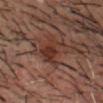This lesion was catalogued during total-body skin photography and was not selected for biopsy.
Automated tile analysis of the lesion measured a detector confidence of about 100 out of 100 that the crop contains a lesion.
A 15 mm close-up tile from a total-body photography series done for melanoma screening.
Approximately 3.5 mm at its widest.
A male subject, roughly 50 years of age.
Imaged with cross-polarized lighting.
From the head or neck.